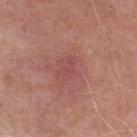Approximately 2.5 mm at its widest. Captured under white-light illumination. The lesion is located on the right lower leg. Cropped from a whole-body photographic skin survey; the tile spans about 15 mm. The patient is a male approximately 65 years of age. An algorithmic analysis of the crop reported an area of roughly 2.5 mm², an outline eccentricity of about 0.8 (0 = round, 1 = elongated), and a shape-asymmetry score of about 0.6 (0 = symmetric).